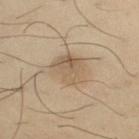No biopsy was performed on this lesion — it was imaged during a full skin examination and was not determined to be concerning. The recorded lesion diameter is about 4 mm. The lesion is on the arm. A male patient aged 38–42. Captured under cross-polarized illumination. A lesion tile, about 15 mm wide, cut from a 3D total-body photograph.